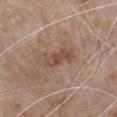notes = imaged on a skin check; not biopsied
illumination = white-light illumination
subject = male, roughly 80 years of age
image source = 15 mm crop, total-body photography
location = the chest